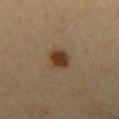Recorded during total-body skin imaging; not selected for excision or biopsy. The total-body-photography lesion software estimated a symmetry-axis asymmetry near 0.15. It also reported roughly 12 lightness units darker than nearby skin and a normalized lesion–skin contrast near 10.5. And it measured internal color variation of about 4 on a 0–10 scale. A male subject, aged 48–52. Cropped from a whole-body photographic skin survey; the tile spans about 15 mm. From the mid back.